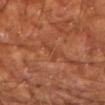Captured during whole-body skin photography for melanoma surveillance; the lesion was not biopsied. The patient is a male aged 63 to 67. Located on the left upper arm. A region of skin cropped from a whole-body photographic capture, roughly 15 mm wide.